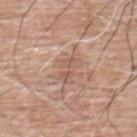TBP lesion metrics: a lesion area of about 7.5 mm², an outline eccentricity of about 0.85 (0 = round, 1 = elongated), and a shape-asymmetry score of about 0.3 (0 = symmetric); a mean CIELAB color near L≈58 a*≈19 b*≈27 and about 7 CIELAB-L* units darker than the surrounding skin | acquisition: ~15 mm crop, total-body skin-cancer survey | location: the back | patient: male, aged 58–62.